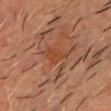This lesion was catalogued during total-body skin photography and was not selected for biopsy. The tile uses cross-polarized illumination. A male patient in their 60s. Measured at roughly 3 mm in maximum diameter. A 15 mm close-up extracted from a 3D total-body photography capture. From the head or neck.